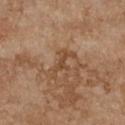{
  "image": {
    "source": "total-body photography crop",
    "field_of_view_mm": 15
  },
  "site": "chest",
  "lesion_size": {
    "long_diameter_mm_approx": 4.0
  },
  "patient": {
    "sex": "female",
    "age_approx": 65
  },
  "lighting": "white-light"
}